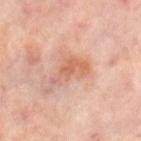This lesion was catalogued during total-body skin photography and was not selected for biopsy.